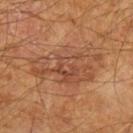Impression: The lesion was photographed on a routine skin check and not biopsied; there is no pathology result. Background: This is a cross-polarized tile. The recorded lesion diameter is about 8 mm. Automated tile analysis of the lesion measured an average lesion color of about L≈47 a*≈22 b*≈32 (CIELAB), roughly 7 lightness units darker than nearby skin, and a normalized border contrast of about 5.5. It also reported border irregularity of about 5.5 on a 0–10 scale, a within-lesion color-variation index near 5.5/10, and peripheral color asymmetry of about 2. This image is a 15 mm lesion crop taken from a total-body photograph. The subject is a male about 60 years old. The lesion is on the left forearm.